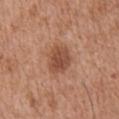| field | value |
|---|---|
| follow-up | total-body-photography surveillance lesion; no biopsy |
| subject | male, roughly 75 years of age |
| anatomic site | the abdomen |
| acquisition | ~15 mm tile from a whole-body skin photo |
| lesion diameter | about 3.5 mm |
| lighting | white-light |
| automated lesion analysis | an average lesion color of about L≈49 a*≈24 b*≈31 (CIELAB), roughly 11 lightness units darker than nearby skin, and a lesion-to-skin contrast of about 8 (normalized; higher = more distinct); a nevus-likeness score of about 80/100 |